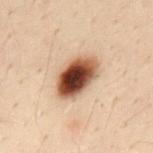Measured at roughly 5.5 mm in maximum diameter. Located on the mid back. A region of skin cropped from a whole-body photographic capture, roughly 15 mm wide. The patient is a male aged approximately 30. The lesion-visualizer software estimated a lesion–skin lightness drop of about 21 and a lesion-to-skin contrast of about 15.5 (normalized; higher = more distinct). It also reported a detector confidence of about 100 out of 100 that the crop contains a lesion. Imaged with cross-polarized lighting.